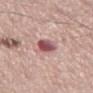follow-up: no biopsy performed (imaged during a skin exam)
site: the leg
image source: 15 mm crop, total-body photography
subject: male, approximately 75 years of age